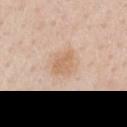Recorded during total-body skin imaging; not selected for excision or biopsy. Cropped from a total-body skin-imaging series; the visible field is about 15 mm. Located on the right upper arm. The tile uses white-light illumination. Automated tile analysis of the lesion measured an area of roughly 6 mm², a shape eccentricity near 0.65, and a shape-asymmetry score of about 0.2 (0 = symmetric). The analysis additionally found a border-irregularity index near 2/10, a color-variation rating of about 1.5/10, and radial color variation of about 0.5. The analysis additionally found an automated nevus-likeness rating near 15 out of 100. The patient is a male roughly 60 years of age.